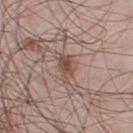This lesion was catalogued during total-body skin photography and was not selected for biopsy.
A 15 mm crop from a total-body photograph taken for skin-cancer surveillance.
The lesion is on the left thigh.
A male patient, aged around 45.
An algorithmic analysis of the crop reported a lesion area of about 4.5 mm², an outline eccentricity of about 0.8 (0 = round, 1 = elongated), and two-axis asymmetry of about 0.35. And it measured a nevus-likeness score of about 85/100 and a detector confidence of about 100 out of 100 that the crop contains a lesion.
Imaged with white-light lighting.